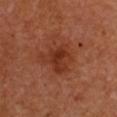Imaged during a routine full-body skin examination; the lesion was not biopsied and no histopathology is available. A female subject approximately 55 years of age. Longest diameter approximately 4 mm. This is a cross-polarized tile. The lesion is located on the front of the torso. A lesion tile, about 15 mm wide, cut from a 3D total-body photograph. Automated tile analysis of the lesion measured a lesion-to-skin contrast of about 7 (normalized; higher = more distinct). The software also gave a border-irregularity index near 4.5/10 and a within-lesion color-variation index near 4/10. It also reported a classifier nevus-likeness of about 5/100.